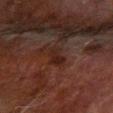Q: Was a biopsy performed?
A: no biopsy performed (imaged during a skin exam)
Q: What is the anatomic site?
A: the left forearm
Q: What lighting was used for the tile?
A: cross-polarized
Q: Lesion size?
A: ~3.5 mm (longest diameter)
Q: What did automated image analysis measure?
A: a lesion color around L≈17 a*≈16 b*≈18 in CIELAB, a lesion–skin lightness drop of about 6, and a lesion-to-skin contrast of about 7.5 (normalized; higher = more distinct); a color-variation rating of about 1.5/10 and radial color variation of about 0.5; an automated nevus-likeness rating near 0 out of 100 and lesion-presence confidence of about 90/100
Q: Who is the patient?
A: male, about 80 years old
Q: How was this image acquired?
A: ~15 mm crop, total-body skin-cancer survey